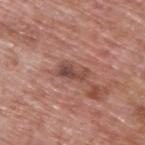<record>
  <biopsy_status>not biopsied; imaged during a skin examination</biopsy_status>
  <patient>
    <sex>male</sex>
    <age_approx>70</age_approx>
  </patient>
  <automated_metrics>
    <cielab_L>47</cielab_L>
    <cielab_a>22</cielab_a>
    <cielab_b>25</cielab_b>
    <vs_skin_darker_L>10.0</vs_skin_darker_L>
    <vs_skin_contrast_norm>7.5</vs_skin_contrast_norm>
    <border_irregularity_0_10>4.5</border_irregularity_0_10>
    <color_variation_0_10>5.5</color_variation_0_10>
    <peripheral_color_asymmetry>2.0</peripheral_color_asymmetry>
    <nevus_likeness_0_100>0</nevus_likeness_0_100>
    <lesion_detection_confidence_0_100>100</lesion_detection_confidence_0_100>
  </automated_metrics>
  <site>upper back</site>
  <lighting>white-light</lighting>
  <image>
    <source>total-body photography crop</source>
    <field_of_view_mm>15</field_of_view_mm>
  </image>
</record>